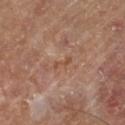subject: male, in their mid- to late 60s | tile lighting: cross-polarized | size: about 2.5 mm | automated lesion analysis: a normalized border contrast of about 6; a border-irregularity index near 5.5/10, a within-lesion color-variation index near 0/10, and peripheral color asymmetry of about 0 | site: the left thigh | acquisition: ~15 mm tile from a whole-body skin photo.